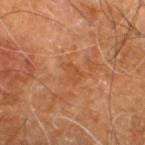follow-up — catalogued during a skin exam; not biopsied
TBP lesion metrics — an area of roughly 3 mm² and a symmetry-axis asymmetry near 0.35; an average lesion color of about L≈48 a*≈25 b*≈38 (CIELAB), a lesion–skin lightness drop of about 5, and a normalized lesion–skin contrast near 5; border irregularity of about 3.5 on a 0–10 scale
patient — male, about 60 years old
site — the right leg
acquisition — total-body-photography crop, ~15 mm field of view